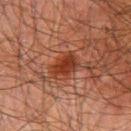* biopsy status: total-body-photography surveillance lesion; no biopsy
* body site: the chest
* size: ≈3.5 mm
* acquisition: total-body-photography crop, ~15 mm field of view
* subject: male, aged approximately 60